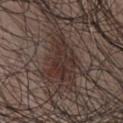notes: total-body-photography surveillance lesion; no biopsy | patient: male, aged 48 to 52 | image: ~15 mm tile from a whole-body skin photo | body site: the front of the torso.